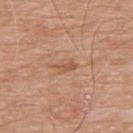{
  "biopsy_status": "not biopsied; imaged during a skin examination",
  "image": {
    "source": "total-body photography crop",
    "field_of_view_mm": 15
  },
  "lesion_size": {
    "long_diameter_mm_approx": 2.5
  },
  "site": "back",
  "patient": {
    "sex": "male",
    "age_approx": 80
  },
  "lighting": "white-light"
}